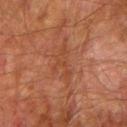Case summary:
- follow-up — total-body-photography surveillance lesion; no biopsy
- site — the right upper arm
- illumination — cross-polarized
- diameter — about 5 mm
- image source — total-body-photography crop, ~15 mm field of view
- subject — male, in their mid- to late 70s
- automated lesion analysis — a mean CIELAB color near L≈37 a*≈22 b*≈29 and about 5 CIELAB-L* units darker than the surrounding skin